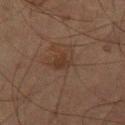<lesion>
  <biopsy_status>not biopsied; imaged during a skin examination</biopsy_status>
  <lighting>cross-polarized</lighting>
  <lesion_size>
    <long_diameter_mm_approx>2.5</long_diameter_mm_approx>
  </lesion_size>
  <patient>
    <sex>male</sex>
    <age_approx>65</age_approx>
  </patient>
  <image>
    <source>total-body photography crop</source>
    <field_of_view_mm>15</field_of_view_mm>
  </image>
  <site>leg</site>
</lesion>